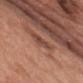Captured during whole-body skin photography for melanoma surveillance; the lesion was not biopsied. The lesion is located on the chest. A 15 mm close-up extracted from a 3D total-body photography capture. A male patient aged approximately 55.